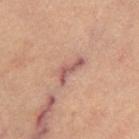workup: catalogued during a skin exam; not biopsied | patient: female, about 65 years old | location: the left leg | image source: total-body-photography crop, ~15 mm field of view | lesion diameter: ~4 mm (longest diameter).